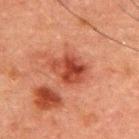Clinical impression: The lesion was photographed on a routine skin check and not biopsied; there is no pathology result. Context: The subject is a male aged 48–52. Imaged with cross-polarized lighting. Cropped from a total-body skin-imaging series; the visible field is about 15 mm. Located on the upper back. An algorithmic analysis of the crop reported a lesion area of about 11 mm², an eccentricity of roughly 0.7, and a shape-asymmetry score of about 0.25 (0 = symmetric). About 4.5 mm across.